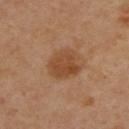Imaged during a routine full-body skin examination; the lesion was not biopsied and no histopathology is available.
Located on the upper back.
The patient is a female approximately 40 years of age.
A 15 mm close-up tile from a total-body photography series done for melanoma screening.